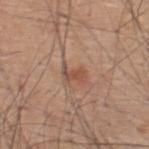{
  "biopsy_status": "not biopsied; imaged during a skin examination",
  "lighting": "white-light",
  "image": {
    "source": "total-body photography crop",
    "field_of_view_mm": 15
  },
  "patient": {
    "sex": "male",
    "age_approx": 60
  },
  "lesion_size": {
    "long_diameter_mm_approx": 3.0
  },
  "site": "upper back"
}